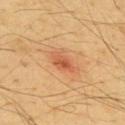Assessment:
This lesion was catalogued during total-body skin photography and was not selected for biopsy.
Acquisition and patient details:
Imaged with cross-polarized lighting. Longest diameter approximately 3 mm. A male subject, in their mid-60s. The lesion is located on the back. The lesion-visualizer software estimated an outline eccentricity of about 0.8 (0 = round, 1 = elongated) and a symmetry-axis asymmetry near 0.25. The software also gave a mean CIELAB color near L≈58 a*≈29 b*≈40, about 10 CIELAB-L* units darker than the surrounding skin, and a normalized border contrast of about 6.5. The analysis additionally found border irregularity of about 2.5 on a 0–10 scale, a within-lesion color-variation index near 5.5/10, and peripheral color asymmetry of about 2. A lesion tile, about 15 mm wide, cut from a 3D total-body photograph.